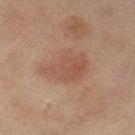Assessment: Captured during whole-body skin photography for melanoma surveillance; the lesion was not biopsied. Background: The lesion is on the left thigh. A 15 mm crop from a total-body photograph taken for skin-cancer surveillance. A female subject approximately 60 years of age.